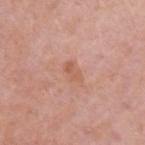notes: total-body-photography surveillance lesion; no biopsy
anatomic site: the arm
subject: female, aged around 40
acquisition: ~15 mm tile from a whole-body skin photo
tile lighting: white-light illumination
lesion size: ~2.5 mm (longest diameter)